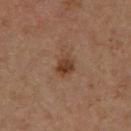Automated image analysis of the tile measured an area of roughly 5 mm² and a symmetry-axis asymmetry near 0.25. And it measured a border-irregularity rating of about 2/10, internal color variation of about 4 on a 0–10 scale, and radial color variation of about 1.5. And it measured an automated nevus-likeness rating near 85 out of 100 and lesion-presence confidence of about 100/100. From the left thigh. A region of skin cropped from a whole-body photographic capture, roughly 15 mm wide. A male patient, approximately 65 years of age. About 2.5 mm across. Imaged with cross-polarized lighting.